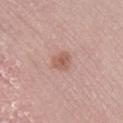Q: Was this lesion biopsied?
A: catalogued during a skin exam; not biopsied